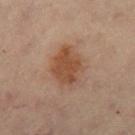{
  "biopsy_status": "not biopsied; imaged during a skin examination",
  "site": "right thigh",
  "image": {
    "source": "total-body photography crop",
    "field_of_view_mm": 15
  },
  "lighting": "cross-polarized",
  "patient": {
    "sex": "female",
    "age_approx": 60
  },
  "lesion_size": {
    "long_diameter_mm_approx": 4.5
  }
}